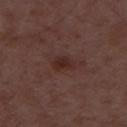Findings:
* notes · no biopsy performed (imaged during a skin exam)
* lighting · white-light
* image · total-body-photography crop, ~15 mm field of view
* automated metrics · a shape eccentricity near 0.75 and a symmetry-axis asymmetry near 0.3; border irregularity of about 2.5 on a 0–10 scale, a color-variation rating of about 1.5/10, and peripheral color asymmetry of about 0.5
* subject · male, about 50 years old
* size · ~2.5 mm (longest diameter)